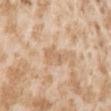The lesion was photographed on a routine skin check and not biopsied; there is no pathology result.
Automated image analysis of the tile measured an eccentricity of roughly 0.9 and a symmetry-axis asymmetry near 0.4. The analysis additionally found border irregularity of about 5.5 on a 0–10 scale, a color-variation rating of about 1/10, and radial color variation of about 0. The software also gave an automated nevus-likeness rating near 0 out of 100 and lesion-presence confidence of about 95/100.
A close-up tile cropped from a whole-body skin photograph, about 15 mm across.
A female patient aged approximately 25.
From the right upper arm.
Measured at roughly 3.5 mm in maximum diameter.
The tile uses white-light illumination.